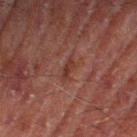| feature | finding |
|---|---|
| follow-up | total-body-photography surveillance lesion; no biopsy |
| site | the right thigh |
| patient | male, approximately 70 years of age |
| acquisition | ~15 mm tile from a whole-body skin photo |